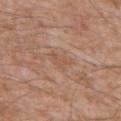Q: How was this image acquired?
A: ~15 mm tile from a whole-body skin photo
Q: What is the anatomic site?
A: the mid back
Q: Who is the patient?
A: male, aged approximately 60
Q: Illumination type?
A: white-light illumination
Q: Lesion size?
A: ~3 mm (longest diameter)